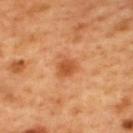Assessment:
Recorded during total-body skin imaging; not selected for excision or biopsy.
Image and clinical context:
Located on the upper back. A male subject aged 48–52. A 15 mm close-up extracted from a 3D total-body photography capture.